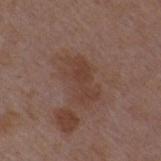The lesion was tiled from a total-body skin photograph and was not biopsied.